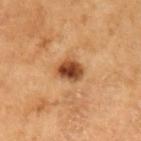{
  "biopsy_status": "not biopsied; imaged during a skin examination",
  "image": {
    "source": "total-body photography crop",
    "field_of_view_mm": 15
  },
  "patient": {
    "sex": "male",
    "age_approx": 60
  },
  "site": "left upper arm",
  "automated_metrics": {
    "cielab_L": 47,
    "cielab_a": 25,
    "cielab_b": 38,
    "vs_skin_darker_L": 17.0,
    "vs_skin_contrast_norm": 11.5,
    "border_irregularity_0_10": 2.0,
    "color_variation_0_10": 7.0,
    "peripheral_color_asymmetry": 2.0
  }
}